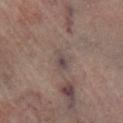{
  "biopsy_status": "not biopsied; imaged during a skin examination",
  "image": {
    "source": "total-body photography crop",
    "field_of_view_mm": 15
  },
  "site": "right lower leg"
}